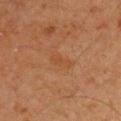Assessment: This lesion was catalogued during total-body skin photography and was not selected for biopsy. Acquisition and patient details: The lesion is on the chest. A male subject aged 58 to 62. A 15 mm crop from a total-body photograph taken for skin-cancer surveillance.